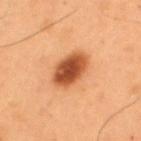{
  "biopsy_status": "not biopsied; imaged during a skin examination",
  "patient": {
    "sex": "male",
    "age_approx": 55
  },
  "lighting": "cross-polarized",
  "lesion_size": {
    "long_diameter_mm_approx": 4.5
  },
  "image": {
    "source": "total-body photography crop",
    "field_of_view_mm": 15
  },
  "site": "upper back",
  "automated_metrics": {
    "vs_skin_darker_L": 18.0,
    "vs_skin_contrast_norm": 11.5,
    "border_irregularity_0_10": 1.5,
    "color_variation_0_10": 5.5
  }
}